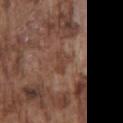body site: the chest | illumination: white-light | acquisition: total-body-photography crop, ~15 mm field of view | automated metrics: a lesion area of about 4 mm², a shape eccentricity near 0.85, and a shape-asymmetry score of about 0.35 (0 = symmetric); a mean CIELAB color near L≈41 a*≈20 b*≈26, about 7 CIELAB-L* units darker than the surrounding skin, and a normalized lesion–skin contrast near 5.5; a border-irregularity rating of about 3.5/10, a color-variation rating of about 1/10, and peripheral color asymmetry of about 0; a detector confidence of about 95 out of 100 that the crop contains a lesion | subject: male, roughly 75 years of age.